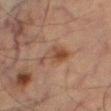biopsy_status: not biopsied; imaged during a skin examination
image:
  source: total-body photography crop
  field_of_view_mm: 15
site: left thigh
automated_metrics:
  shape_asymmetry: 0.45
  border_irregularity_0_10: 5.0
  color_variation_0_10: 5.0
  peripheral_color_asymmetry: 1.5
  nevus_likeness_0_100: 10
patient:
  sex: male
  age_approx: 65
lesion_size:
  long_diameter_mm_approx: 4.5